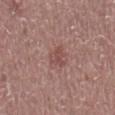Assessment: The lesion was tiled from a total-body skin photograph and was not biopsied. Clinical summary: A male patient, in their 60s. This image is a 15 mm lesion crop taken from a total-body photograph. The lesion-visualizer software estimated an area of roughly 4 mm², an eccentricity of roughly 0.6, and two-axis asymmetry of about 0.4. The analysis additionally found border irregularity of about 4 on a 0–10 scale and peripheral color asymmetry of about 0.5. The lesion is located on the mid back. Measured at roughly 2.5 mm in maximum diameter.